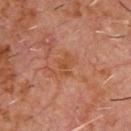This lesion was catalogued during total-body skin photography and was not selected for biopsy.
The patient is a male in their 60s.
Automated image analysis of the tile measured a footprint of about 3.5 mm², an eccentricity of roughly 0.85, and a shape-asymmetry score of about 0.4 (0 = symmetric).
The tile uses cross-polarized illumination.
Measured at roughly 3 mm in maximum diameter.
Located on the chest.
A 15 mm crop from a total-body photograph taken for skin-cancer surveillance.